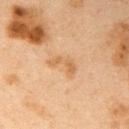Background:
Cropped from a total-body skin-imaging series; the visible field is about 15 mm. A female patient, about 40 years old. Approximately 3.5 mm at its widest. Captured under cross-polarized illumination. The lesion is on the arm.